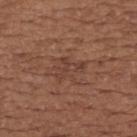* follow-up: total-body-photography surveillance lesion; no biopsy
* illumination: white-light
* patient: female, aged 63 to 67
* lesion diameter: about 4 mm
* site: the upper back
* imaging modality: ~15 mm crop, total-body skin-cancer survey